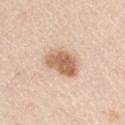<case>
  <patient>
    <sex>male</sex>
    <age_approx>45</age_approx>
  </patient>
  <image>
    <source>total-body photography crop</source>
    <field_of_view_mm>15</field_of_view_mm>
  </image>
  <site>arm</site>
  <lighting>white-light</lighting>
</case>